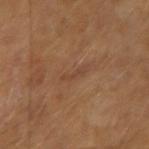The subject is aged 53 to 57. The lesion is on the right upper arm. A roughly 15 mm field-of-view crop from a total-body skin photograph.